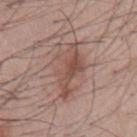| feature | finding |
|---|---|
| notes | no biopsy performed (imaged during a skin exam) |
| image | 15 mm crop, total-body photography |
| subject | male, about 55 years old |
| body site | the front of the torso |